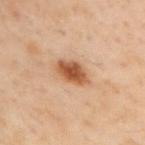{"biopsy_status": "not biopsied; imaged during a skin examination", "image": {"source": "total-body photography crop", "field_of_view_mm": 15}, "site": "right upper arm", "patient": {"sex": "male", "age_approx": 55}, "lighting": "cross-polarized"}